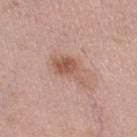Impression:
Imaged during a routine full-body skin examination; the lesion was not biopsied and no histopathology is available.
Image and clinical context:
This image is a 15 mm lesion crop taken from a total-body photograph. A female patient in their mid- to late 60s. From the right thigh. The tile uses white-light illumination.